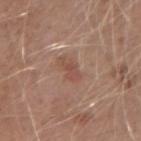Q: Was this lesion biopsied?
A: catalogued during a skin exam; not biopsied
Q: Who is the patient?
A: male, in their mid-20s
Q: How was the tile lit?
A: white-light
Q: What kind of image is this?
A: total-body-photography crop, ~15 mm field of view
Q: Lesion location?
A: the left forearm
Q: What is the lesion's diameter?
A: about 2.5 mm